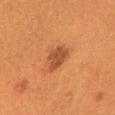Case summary:
– biopsy status: total-body-photography surveillance lesion; no biopsy
– image source: total-body-photography crop, ~15 mm field of view
– diameter: ≈3.5 mm
– subject: female, aged approximately 40
– illumination: cross-polarized illumination
– automated metrics: an area of roughly 6.5 mm², an outline eccentricity of about 0.8 (0 = round, 1 = elongated), and two-axis asymmetry of about 0.25; a lesion color around L≈40 a*≈24 b*≈33 in CIELAB, a lesion–skin lightness drop of about 9, and a normalized border contrast of about 8; a border-irregularity rating of about 2.5/10, a color-variation rating of about 2/10, and peripheral color asymmetry of about 0.5; a detector confidence of about 100 out of 100 that the crop contains a lesion
– site: the chest